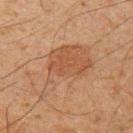Background: On the left upper arm. A male patient, aged 33–37. A lesion tile, about 15 mm wide, cut from a 3D total-body photograph. The lesion-visualizer software estimated a lesion color around L≈40 a*≈17 b*≈27 in CIELAB and a normalized lesion–skin contrast near 5. The analysis additionally found a border-irregularity index near 6/10, a color-variation rating of about 3/10, and radial color variation of about 1. And it measured a nevus-likeness score of about 70/100 and lesion-presence confidence of about 100/100. Approximately 8.5 mm at its widest. Captured under cross-polarized illumination.